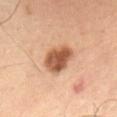The lesion was tiled from a total-body skin photograph and was not biopsied.
The lesion is located on the lower back.
Imaged with cross-polarized lighting.
A male patient, in their 40s.
Automated image analysis of the tile measured an area of roughly 10 mm², a shape eccentricity near 0.6, and a shape-asymmetry score of about 0.2 (0 = symmetric). And it measured a mean CIELAB color near L≈54 a*≈23 b*≈34, roughly 16 lightness units darker than nearby skin, and a normalized border contrast of about 10.5.
Cropped from a whole-body photographic skin survey; the tile spans about 15 mm.
The lesion's longest dimension is about 4 mm.